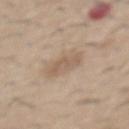Q: Was this lesion biopsied?
A: no biopsy performed (imaged during a skin exam)
Q: What kind of image is this?
A: ~15 mm crop, total-body skin-cancer survey
Q: How large is the lesion?
A: about 3 mm
Q: What is the anatomic site?
A: the abdomen
Q: What are the patient's age and sex?
A: male, approximately 60 years of age